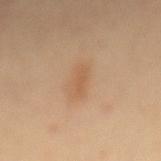Automated tile analysis of the lesion measured roughly 7 lightness units darker than nearby skin and a normalized lesion–skin contrast near 5.5. And it measured a border-irregularity index near 3/10 and radial color variation of about 0.
A region of skin cropped from a whole-body photographic capture, roughly 15 mm wide.
The lesion is located on the mid back.
This is a cross-polarized tile.
A patient approximately 70 years of age.
About 3 mm across.